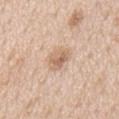Part of a total-body skin-imaging series; this lesion was reviewed on a skin check and was not flagged for biopsy.
Cropped from a total-body skin-imaging series; the visible field is about 15 mm.
From the chest.
The subject is a male in their mid- to late 60s.
This is a white-light tile.
Measured at roughly 3.5 mm in maximum diameter.
The total-body-photography lesion software estimated a footprint of about 6 mm², an eccentricity of roughly 0.75, and a shape-asymmetry score of about 0.25 (0 = symmetric).